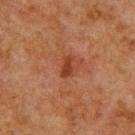<lesion>
<biopsy_status>not biopsied; imaged during a skin examination</biopsy_status>
<patient>
  <sex>male</sex>
  <age_approx>60</age_approx>
</patient>
<automated_metrics>
  <area_mm2_approx>3.0</area_mm2_approx>
  <eccentricity>0.85</eccentricity>
  <border_irregularity_0_10>3.5</border_irregularity_0_10>
  <color_variation_0_10>0.5</color_variation_0_10>
  <peripheral_color_asymmetry>0.0</peripheral_color_asymmetry>
</automated_metrics>
<lighting>cross-polarized</lighting>
<lesion_size>
  <long_diameter_mm_approx>2.5</long_diameter_mm_approx>
</lesion_size>
<site>upper back</site>
<image>
  <source>total-body photography crop</source>
  <field_of_view_mm>15</field_of_view_mm>
</image>
</lesion>